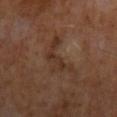Assessment: Imaged during a routine full-body skin examination; the lesion was not biopsied and no histopathology is available. Clinical summary: This is a cross-polarized tile. A 15 mm close-up tile from a total-body photography series done for melanoma screening. A male subject aged 63 to 67.